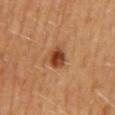This lesion was catalogued during total-body skin photography and was not selected for biopsy. From the mid back. A 15 mm close-up tile from a total-body photography series done for melanoma screening. Measured at roughly 3 mm in maximum diameter. A female patient. The tile uses cross-polarized illumination.